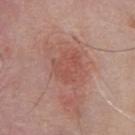| key | value |
|---|---|
| illumination | white-light illumination |
| automated lesion analysis | a border-irregularity index near 3.5/10, a within-lesion color-variation index near 2.5/10, and a peripheral color-asymmetry measure near 1; a classifier nevus-likeness of about 35/100 and lesion-presence confidence of about 100/100 |
| subject | male, in their 80s |
| diameter | about 3.5 mm |
| site | the chest |
| image source | ~15 mm crop, total-body skin-cancer survey |